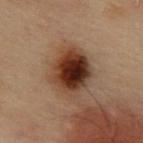Captured during whole-body skin photography for melanoma surveillance; the lesion was not biopsied. From the abdomen. A male patient aged around 55. A roughly 15 mm field-of-view crop from a total-body skin photograph.